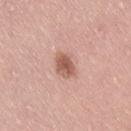Notes:
• notes: imaged on a skin check; not biopsied
• acquisition: 15 mm crop, total-body photography
• illumination: white-light illumination
• subject: male, in their 40s
• size: ~3 mm (longest diameter)
• image-analysis metrics: border irregularity of about 2 on a 0–10 scale, a color-variation rating of about 3/10, and peripheral color asymmetry of about 1
• location: the back